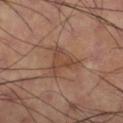The lesion was photographed on a routine skin check and not biopsied; there is no pathology result. From the right thigh. A close-up tile cropped from a whole-body skin photograph, about 15 mm across. The tile uses cross-polarized illumination. The patient is a male aged 58 to 62.